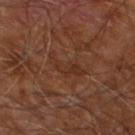Q: Where on the body is the lesion?
A: the right leg
Q: What is the lesion's diameter?
A: ~4.5 mm (longest diameter)
Q: How was the tile lit?
A: cross-polarized illumination
Q: What are the patient's age and sex?
A: male, in their 60s
Q: Automated lesion metrics?
A: an area of roughly 6 mm² and a shape eccentricity near 0.9; a lesion color around L≈30 a*≈19 b*≈27 in CIELAB, roughly 5 lightness units darker than nearby skin, and a normalized lesion–skin contrast near 5.5; an automated nevus-likeness rating near 0 out of 100 and lesion-presence confidence of about 65/100
Q: How was this image acquired?
A: total-body-photography crop, ~15 mm field of view